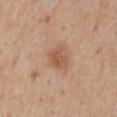The lesion was tiled from a total-body skin photograph and was not biopsied. Approximately 3 mm at its widest. The lesion is located on the back. A region of skin cropped from a whole-body photographic capture, roughly 15 mm wide. The subject is a male aged 53–57. The total-body-photography lesion software estimated a lesion area of about 5.5 mm², an outline eccentricity of about 0.6 (0 = round, 1 = elongated), and two-axis asymmetry of about 0.25. The analysis additionally found a lesion color around L≈55 a*≈21 b*≈32 in CIELAB, a lesion–skin lightness drop of about 9, and a normalized lesion–skin contrast near 6.5. The software also gave a border-irregularity index near 2.5/10 and radial color variation of about 1.